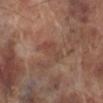Q: Was a biopsy performed?
A: imaged on a skin check; not biopsied
Q: Who is the patient?
A: male, aged 68 to 72
Q: What is the imaging modality?
A: ~15 mm crop, total-body skin-cancer survey
Q: What is the anatomic site?
A: the left lower leg